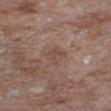This lesion was catalogued during total-body skin photography and was not selected for biopsy.
Imaged with white-light lighting.
Automated tile analysis of the lesion measured an average lesion color of about L≈47 a*≈18 b*≈25 (CIELAB) and roughly 6 lightness units darker than nearby skin. It also reported a border-irregularity rating of about 2/10, a within-lesion color-variation index near 2.5/10, and peripheral color asymmetry of about 1.
On the chest.
A close-up tile cropped from a whole-body skin photograph, about 15 mm across.
A female patient in their mid- to late 70s.
About 2.5 mm across.